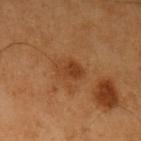The lesion was photographed on a routine skin check and not biopsied; there is no pathology result. A roughly 15 mm field-of-view crop from a total-body skin photograph. A male patient approximately 60 years of age. Automated image analysis of the tile measured a border-irregularity index near 2.5/10, a color-variation rating of about 4/10, and a peripheral color-asymmetry measure near 1.5. And it measured lesion-presence confidence of about 100/100. From the left upper arm. This is a cross-polarized tile.